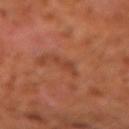Impression: Recorded during total-body skin imaging; not selected for excision or biopsy. Acquisition and patient details: Located on the left forearm. Captured under cross-polarized illumination. A 15 mm crop from a total-body photograph taken for skin-cancer surveillance. A male patient, approximately 60 years of age. Automated image analysis of the tile measured a footprint of about 4 mm², a shape eccentricity near 0.85, and a shape-asymmetry score of about 0.35 (0 = symmetric). It also reported border irregularity of about 4 on a 0–10 scale, a color-variation rating of about 1/10, and radial color variation of about 0.5. And it measured a nevus-likeness score of about 0/100 and a lesion-detection confidence of about 95/100.